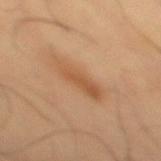No biopsy was performed on this lesion — it was imaged during a full skin examination and was not determined to be concerning. Located on the mid back. This image is a 15 mm lesion crop taken from a total-body photograph. A male patient in their mid- to late 50s.